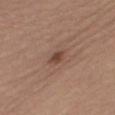No biopsy was performed on this lesion — it was imaged during a full skin examination and was not determined to be concerning. Captured under white-light illumination. A female patient aged 63–67. The lesion is on the right thigh. A lesion tile, about 15 mm wide, cut from a 3D total-body photograph.